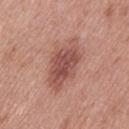Recorded during total-body skin imaging; not selected for excision or biopsy.
On the left thigh.
Cropped from a whole-body photographic skin survey; the tile spans about 15 mm.
The lesion's longest dimension is about 7 mm.
The lesion-visualizer software estimated an area of roughly 17 mm², a shape eccentricity near 0.85, and a symmetry-axis asymmetry near 0.2. And it measured an average lesion color of about L≈52 a*≈24 b*≈25 (CIELAB), about 11 CIELAB-L* units darker than the surrounding skin, and a normalized lesion–skin contrast near 8. And it measured a border-irregularity index near 3/10, internal color variation of about 4 on a 0–10 scale, and radial color variation of about 1.5.
The patient is a female aged 38 to 42.
Imaged with white-light lighting.